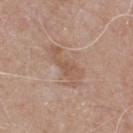{
  "biopsy_status": "not biopsied; imaged during a skin examination",
  "patient": {
    "sex": "male",
    "age_approx": 75
  },
  "lighting": "white-light",
  "image": {
    "source": "total-body photography crop",
    "field_of_view_mm": 15
  },
  "site": "chest",
  "automated_metrics": {
    "area_mm2_approx": 8.5,
    "cielab_L": 55,
    "cielab_a": 18,
    "cielab_b": 28,
    "vs_skin_darker_L": 7.0,
    "lesion_detection_confidence_0_100": 100
  }
}